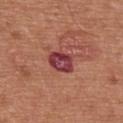| feature | finding |
|---|---|
| notes | total-body-photography surveillance lesion; no biopsy |
| illumination | white-light illumination |
| lesion diameter | about 3.5 mm |
| automated lesion analysis | an area of roughly 7.5 mm², an eccentricity of roughly 0.55, and a symmetry-axis asymmetry near 0.2; a mean CIELAB color near L≈41 a*≈32 b*≈22, about 13 CIELAB-L* units darker than the surrounding skin, and a lesion-to-skin contrast of about 11 (normalized; higher = more distinct); a nevus-likeness score of about 0/100 and a detector confidence of about 100 out of 100 that the crop contains a lesion |
| patient | male, aged around 65 |
| site | the back |
| image source | ~15 mm crop, total-body skin-cancer survey |